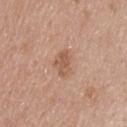Clinical impression:
The lesion was tiled from a total-body skin photograph and was not biopsied.
Context:
This image is a 15 mm lesion crop taken from a total-body photograph. The recorded lesion diameter is about 3 mm. A male subject, aged around 45. The total-body-photography lesion software estimated a footprint of about 4.5 mm², a shape eccentricity near 0.8, and two-axis asymmetry of about 0.3. And it measured an average lesion color of about L≈56 a*≈20 b*≈31 (CIELAB), a lesion–skin lightness drop of about 9, and a lesion-to-skin contrast of about 6.5 (normalized; higher = more distinct). And it measured a classifier nevus-likeness of about 10/100. On the back. The tile uses white-light illumination.